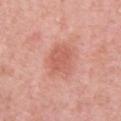Context: The lesion is located on the back. A roughly 15 mm field-of-view crop from a total-body skin photograph. Imaged with white-light lighting. The patient is a male in their 50s.